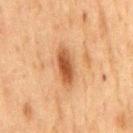| field | value |
|---|---|
| biopsy status | no biopsy performed (imaged during a skin exam) |
| acquisition | total-body-photography crop, ~15 mm field of view |
| anatomic site | the chest |
| patient | male, aged around 75 |
| lesion diameter | ~5 mm (longest diameter) |
| lighting | cross-polarized |